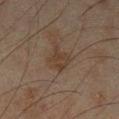Clinical impression:
Imaged during a routine full-body skin examination; the lesion was not biopsied and no histopathology is available.
Acquisition and patient details:
Approximately 3 mm at its widest. From the leg. A male subject aged around 45. A 15 mm close-up tile from a total-body photography series done for melanoma screening.